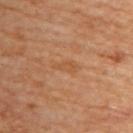Clinical impression:
Captured during whole-body skin photography for melanoma surveillance; the lesion was not biopsied.
Clinical summary:
From the back. A region of skin cropped from a whole-body photographic capture, roughly 15 mm wide. The subject is a female in their mid- to late 60s.